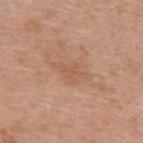{
  "biopsy_status": "not biopsied; imaged during a skin examination",
  "site": "upper back",
  "patient": {
    "sex": "female",
    "age_approx": 40
  },
  "image": {
    "source": "total-body photography crop",
    "field_of_view_mm": 15
  },
  "automated_metrics": {
    "area_mm2_approx": 5.0,
    "eccentricity": 0.85,
    "shape_asymmetry": 0.35,
    "cielab_L": 57,
    "cielab_a": 21,
    "cielab_b": 32,
    "vs_skin_darker_L": 6.0,
    "vs_skin_contrast_norm": 4.5,
    "border_irregularity_0_10": 4.5,
    "color_variation_0_10": 2.0,
    "peripheral_color_asymmetry": 1.0,
    "nevus_likeness_0_100": 0
  },
  "lesion_size": {
    "long_diameter_mm_approx": 4.0
  }
}